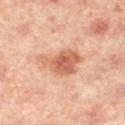– notes · no biopsy performed (imaged during a skin exam)
– illumination · cross-polarized illumination
– patient · female, aged approximately 40
– size · about 5.5 mm
– location · the left lower leg
– acquisition · ~15 mm crop, total-body skin-cancer survey
– TBP lesion metrics · a border-irregularity index near 4/10, internal color variation of about 3.5 on a 0–10 scale, and radial color variation of about 1